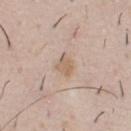{"biopsy_status": "not biopsied; imaged during a skin examination", "lighting": "white-light", "image": {"source": "total-body photography crop", "field_of_view_mm": 15}, "site": "chest", "lesion_size": {"long_diameter_mm_approx": 2.5}, "patient": {"sex": "male", "age_approx": 40}, "automated_metrics": {"cielab_L": 62, "cielab_a": 15, "cielab_b": 29}}